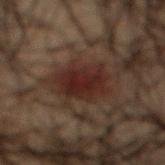This lesion was catalogued during total-body skin photography and was not selected for biopsy.
The lesion is on the mid back.
This is a cross-polarized tile.
The subject is a male aged 48 to 52.
A roughly 15 mm field-of-view crop from a total-body skin photograph.